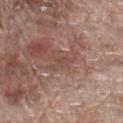Clinical impression:
No biopsy was performed on this lesion — it was imaged during a full skin examination and was not determined to be concerning.
Background:
This is a white-light tile. An algorithmic analysis of the crop reported a mean CIELAB color near L≈47 a*≈19 b*≈24, a lesion–skin lightness drop of about 6, and a normalized border contrast of about 5. Cropped from a total-body skin-imaging series; the visible field is about 15 mm. A male subject, aged approximately 70. From the leg.